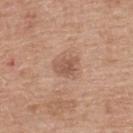biopsy_status: not biopsied; imaged during a skin examination
patient:
  sex: female
  age_approx: 60
site: upper back
lighting: white-light
lesion_size:
  long_diameter_mm_approx: 3.0
image:
  source: total-body photography crop
  field_of_view_mm: 15